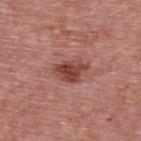Assessment:
This lesion was catalogued during total-body skin photography and was not selected for biopsy.
Context:
A 15 mm close-up extracted from a 3D total-body photography capture. Automated tile analysis of the lesion measured a footprint of about 7 mm², a shape eccentricity near 0.75, and two-axis asymmetry of about 0.35. It also reported a lesion color around L≈44 a*≈26 b*≈26 in CIELAB, roughly 12 lightness units darker than nearby skin, and a normalized lesion–skin contrast near 9.5. The analysis additionally found a border-irregularity rating of about 3.5/10, internal color variation of about 4 on a 0–10 scale, and radial color variation of about 1.5. The software also gave a classifier nevus-likeness of about 85/100. Longest diameter approximately 4 mm. A male patient aged around 75. The lesion is located on the upper back.